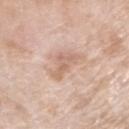Part of a total-body skin-imaging series; this lesion was reviewed on a skin check and was not flagged for biopsy. Located on the right upper arm. A female patient aged around 75. The tile uses white-light illumination. Approximately 4 mm at its widest. A region of skin cropped from a whole-body photographic capture, roughly 15 mm wide.